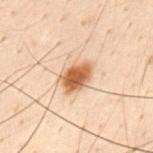Clinical impression: The lesion was photographed on a routine skin check and not biopsied; there is no pathology result. Acquisition and patient details: A 15 mm close-up extracted from a 3D total-body photography capture. Located on the mid back. An algorithmic analysis of the crop reported border irregularity of about 2 on a 0–10 scale, a within-lesion color-variation index near 4/10, and a peripheral color-asymmetry measure near 1. The software also gave a nevus-likeness score of about 100/100. The subject is a male in their 30s. Imaged with cross-polarized lighting.